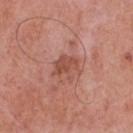Imaged during a routine full-body skin examination; the lesion was not biopsied and no histopathology is available.
This is a white-light tile.
A male subject, aged 68–72.
Approximately 3 mm at its widest.
From the chest.
This image is a 15 mm lesion crop taken from a total-body photograph.